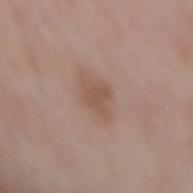Part of a total-body skin-imaging series; this lesion was reviewed on a skin check and was not flagged for biopsy. A 15 mm close-up extracted from a 3D total-body photography capture. The lesion is located on the mid back. This is a white-light tile. Automated image analysis of the tile measured an area of roughly 8 mm² and two-axis asymmetry of about 0.25. It also reported a border-irregularity index near 3/10, internal color variation of about 1.5 on a 0–10 scale, and a peripheral color-asymmetry measure near 0.5. The analysis additionally found an automated nevus-likeness rating near 0 out of 100 and lesion-presence confidence of about 100/100. The lesion's longest dimension is about 4.5 mm. The subject is a female aged 68 to 72.